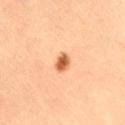Part of a total-body skin-imaging series; this lesion was reviewed on a skin check and was not flagged for biopsy. The subject is a female about 50 years old. An algorithmic analysis of the crop reported a shape eccentricity near 0.75 and a symmetry-axis asymmetry near 0.25. Cropped from a whole-body photographic skin survey; the tile spans about 15 mm.